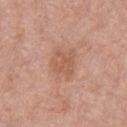Findings:
– follow-up — no biopsy performed (imaged during a skin exam)
– tile lighting — white-light illumination
– size — about 3.5 mm
– imaging modality — ~15 mm tile from a whole-body skin photo
– anatomic site — the chest
– subject — male, approximately 55 years of age